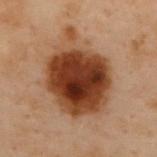Case summary:
- follow-up — imaged on a skin check; not biopsied
- body site — the upper back
- tile lighting — cross-polarized
- subject — female, roughly 60 years of age
- image source — total-body-photography crop, ~15 mm field of view
- diameter — ~9 mm (longest diameter)